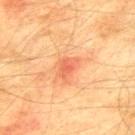Q: Was a biopsy performed?
A: no biopsy performed (imaged during a skin exam)
Q: Where on the body is the lesion?
A: the mid back
Q: Patient demographics?
A: male, in their mid- to late 70s
Q: Illumination type?
A: cross-polarized
Q: What kind of image is this?
A: total-body-photography crop, ~15 mm field of view